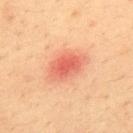The lesion was tiled from a total-body skin photograph and was not biopsied. A lesion tile, about 15 mm wide, cut from a 3D total-body photograph. On the upper back. A male patient, aged 33–37. The recorded lesion diameter is about 4.5 mm. The tile uses cross-polarized illumination.